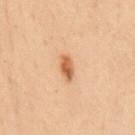follow-up: imaged on a skin check; not biopsied
lesion diameter: ≈3.5 mm
patient: female, aged approximately 50
lighting: cross-polarized
site: the mid back
imaging modality: ~15 mm crop, total-body skin-cancer survey